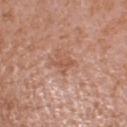The subject is a female approximately 35 years of age. A 15 mm close-up tile from a total-body photography series done for melanoma screening. Automated image analysis of the tile measured a footprint of about 2 mm², an outline eccentricity of about 0.95 (0 = round, 1 = elongated), and a shape-asymmetry score of about 0.45 (0 = symmetric). The software also gave a lesion–skin lightness drop of about 8. And it measured a border-irregularity index near 5/10 and a peripheral color-asymmetry measure near 0. The software also gave a nevus-likeness score of about 0/100 and a lesion-detection confidence of about 100/100. Located on the head or neck.